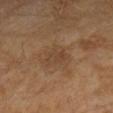Q: Was a biopsy performed?
A: no biopsy performed (imaged during a skin exam)
Q: What did automated image analysis measure?
A: a mean CIELAB color near L≈38 a*≈16 b*≈27, roughly 5 lightness units darker than nearby skin, and a lesion-to-skin contrast of about 5 (normalized; higher = more distinct); border irregularity of about 2.5 on a 0–10 scale, internal color variation of about 2 on a 0–10 scale, and peripheral color asymmetry of about 0.5
Q: Illumination type?
A: cross-polarized illumination
Q: Who is the patient?
A: female, about 60 years old
Q: Where on the body is the lesion?
A: the left forearm
Q: What kind of image is this?
A: total-body-photography crop, ~15 mm field of view